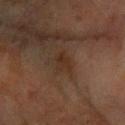Impression: The lesion was tiled from a total-body skin photograph and was not biopsied. Clinical summary: Cropped from a whole-body photographic skin survey; the tile spans about 15 mm. About 2.5 mm across. On the arm. A female patient aged around 70. This is a cross-polarized tile. The lesion-visualizer software estimated a lesion-to-skin contrast of about 6 (normalized; higher = more distinct).